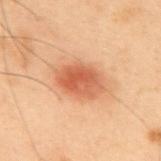Findings:
• workup: catalogued during a skin exam; not biopsied
• anatomic site: the back
• image: total-body-photography crop, ~15 mm field of view
• subject: male, aged approximately 55
• tile lighting: cross-polarized